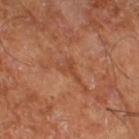{"site": "right thigh", "image": {"source": "total-body photography crop", "field_of_view_mm": 15}, "patient": {"age_approx": 65}}